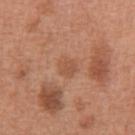<tbp_lesion>
<biopsy_status>not biopsied; imaged during a skin examination</biopsy_status>
</tbp_lesion>